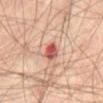Clinical impression: Imaged during a routine full-body skin examination; the lesion was not biopsied and no histopathology is available. Acquisition and patient details: Longest diameter approximately 3 mm. This is a cross-polarized tile. A lesion tile, about 15 mm wide, cut from a 3D total-body photograph. The subject is a male aged 63–67. Automated image analysis of the tile measured a footprint of about 4 mm² and a symmetry-axis asymmetry near 0.3. The software also gave an average lesion color of about L≈54 a*≈31 b*≈28 (CIELAB) and a lesion–skin lightness drop of about 15. And it measured border irregularity of about 2.5 on a 0–10 scale and internal color variation of about 8.5 on a 0–10 scale. And it measured a classifier nevus-likeness of about 10/100 and lesion-presence confidence of about 100/100. The lesion is on the abdomen.